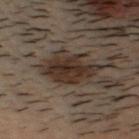Image and clinical context:
The lesion is located on the head or neck. Cropped from a whole-body photographic skin survey; the tile spans about 15 mm. This is a cross-polarized tile. The subject is a male aged around 30.